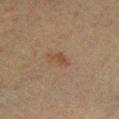Imaged during a routine full-body skin examination; the lesion was not biopsied and no histopathology is available. A male subject, aged around 50. Imaged with cross-polarized lighting. Cropped from a whole-body photographic skin survey; the tile spans about 15 mm. The recorded lesion diameter is about 2.5 mm. Located on the right lower leg.